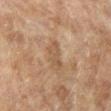This lesion was catalogued during total-body skin photography and was not selected for biopsy.
A female patient, aged 58–62.
A region of skin cropped from a whole-body photographic capture, roughly 15 mm wide.
Captured under cross-polarized illumination.
The lesion-visualizer software estimated border irregularity of about 4 on a 0–10 scale and radial color variation of about 0.5. The analysis additionally found an automated nevus-likeness rating near 0 out of 100 and a detector confidence of about 100 out of 100 that the crop contains a lesion.
Located on the right lower leg.
About 3.5 mm across.